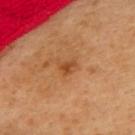Impression: Recorded during total-body skin imaging; not selected for excision or biopsy. Clinical summary: A roughly 15 mm field-of-view crop from a total-body skin photograph. The subject is a female in their mid-30s. The total-body-photography lesion software estimated a lesion area of about 2 mm², an outline eccentricity of about 0.65 (0 = round, 1 = elongated), and a shape-asymmetry score of about 0.45 (0 = symmetric). And it measured a mean CIELAB color near L≈46 a*≈26 b*≈40 and a normalized border contrast of about 7.5. It also reported internal color variation of about 0 on a 0–10 scale and a peripheral color-asymmetry measure near 0. The analysis additionally found an automated nevus-likeness rating near 45 out of 100 and a detector confidence of about 100 out of 100 that the crop contains a lesion. Captured under cross-polarized illumination. The lesion is located on the upper back.